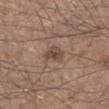{"biopsy_status": "not biopsied; imaged during a skin examination", "lighting": "white-light", "site": "leg", "lesion_size": {"long_diameter_mm_approx": 2.5}, "image": {"source": "total-body photography crop", "field_of_view_mm": 15}, "automated_metrics": {"area_mm2_approx": 4.5, "eccentricity": 0.7, "shape_asymmetry": 0.3, "cielab_L": 46, "cielab_a": 16, "cielab_b": 25, "vs_skin_darker_L": 9.0, "vs_skin_contrast_norm": 7.0}, "patient": {"sex": "male", "age_approx": 45}}